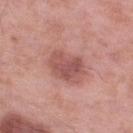follow-up: catalogued during a skin exam; not biopsied
site: the leg
imaging modality: total-body-photography crop, ~15 mm field of view
diameter: about 4 mm
subject: male, aged 53–57
tile lighting: white-light illumination